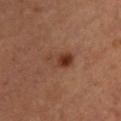Findings:
- workup — catalogued during a skin exam; not biopsied
- tile lighting — cross-polarized illumination
- site — the chest
- imaging modality — total-body-photography crop, ~15 mm field of view
- patient — female, aged 43 to 47
- diameter — ~3.5 mm (longest diameter)